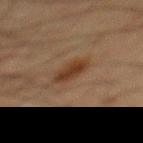Recorded during total-body skin imaging; not selected for excision or biopsy. The subject is a male roughly 60 years of age. Longest diameter approximately 3.5 mm. This is a cross-polarized tile. A close-up tile cropped from a whole-body skin photograph, about 15 mm across. From the back.